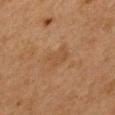Impression: This lesion was catalogued during total-body skin photography and was not selected for biopsy. Acquisition and patient details: The lesion is located on the mid back. The patient is a female aged approximately 40. About 3 mm across. This is a cross-polarized tile. This image is a 15 mm lesion crop taken from a total-body photograph.